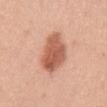The lesion was photographed on a routine skin check and not biopsied; there is no pathology result.
Captured under white-light illumination.
Cropped from a whole-body photographic skin survey; the tile spans about 15 mm.
On the right upper arm.
The total-body-photography lesion software estimated an average lesion color of about L≈59 a*≈26 b*≈32 (CIELAB), roughly 14 lightness units darker than nearby skin, and a normalized border contrast of about 9.
A female subject, aged 33 to 37.